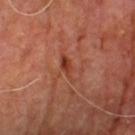Impression:
No biopsy was performed on this lesion — it was imaged during a full skin examination and was not determined to be concerning.
Clinical summary:
This image is a 15 mm lesion crop taken from a total-body photograph. The lesion is located on the back. Automated tile analysis of the lesion measured an area of roughly 3.5 mm², an outline eccentricity of about 0.95 (0 = round, 1 = elongated), and two-axis asymmetry of about 0.3. It also reported a peripheral color-asymmetry measure near 0. Approximately 4 mm at its widest. This is a cross-polarized tile. A male subject roughly 60 years of age.